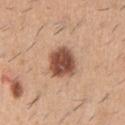{"biopsy_status": "not biopsied; imaged during a skin examination", "patient": {"sex": "male", "age_approx": 60}, "site": "arm", "image": {"source": "total-body photography crop", "field_of_view_mm": 15}}